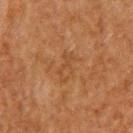| feature | finding |
|---|---|
| lesion size | about 3.5 mm |
| subject | male, aged approximately 50 |
| body site | the upper back |
| tile lighting | cross-polarized |
| image source | ~15 mm tile from a whole-body skin photo |